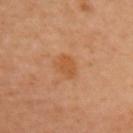Recorded during total-body skin imaging; not selected for excision or biopsy. Cropped from a total-body skin-imaging series; the visible field is about 15 mm. A female subject about 60 years old. Longest diameter approximately 3 mm. The lesion is on the chest. The lesion-visualizer software estimated an area of roughly 5.5 mm², a shape eccentricity near 0.55, and a shape-asymmetry score of about 0.15 (0 = symmetric). The software also gave a mean CIELAB color near L≈46 a*≈22 b*≈35, roughly 6 lightness units darker than nearby skin, and a lesion-to-skin contrast of about 6 (normalized; higher = more distinct).